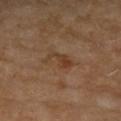  biopsy_status: not biopsied; imaged during a skin examination
  lesion_size:
    long_diameter_mm_approx: 3.5
  patient:
    sex: female
    age_approx: 70
  lighting: cross-polarized
  image:
    source: total-body photography crop
    field_of_view_mm: 15
  automated_metrics:
    vs_skin_darker_L: 7.0
    vs_skin_contrast_norm: 6.5
    nevus_likeness_0_100: 5
    lesion_detection_confidence_0_100: 100
  site: arm